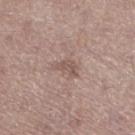  biopsy_status: not biopsied; imaged during a skin examination
  patient:
    sex: female
    age_approx: 50
  image:
    source: total-body photography crop
    field_of_view_mm: 15
  site: left lower leg
  automated_metrics:
    area_mm2_approx: 3.0
    eccentricity: 0.85
    shape_asymmetry: 0.5
    nevus_likeness_0_100: 0
    lesion_detection_confidence_0_100: 100